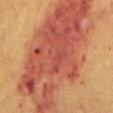Imaged during a routine full-body skin examination; the lesion was not biopsied and no histopathology is available.
A close-up tile cropped from a whole-body skin photograph, about 15 mm across.
Captured under cross-polarized illumination.
Located on the mid back.
A female patient approximately 55 years of age.